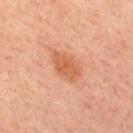Clinical impression: Recorded during total-body skin imaging; not selected for excision or biopsy. Acquisition and patient details: The recorded lesion diameter is about 4 mm. A male patient in their 30s. Captured under cross-polarized illumination. This image is a 15 mm lesion crop taken from a total-body photograph. The lesion is on the upper back.